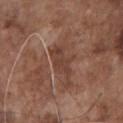Clinical impression: This lesion was catalogued during total-body skin photography and was not selected for biopsy. Acquisition and patient details: The lesion is on the chest. An algorithmic analysis of the crop reported a lesion area of about 7.5 mm², a shape eccentricity near 0.9, and a shape-asymmetry score of about 0.35 (0 = symmetric). The subject is a male about 75 years old. Cropped from a total-body skin-imaging series; the visible field is about 15 mm. This is a white-light tile.